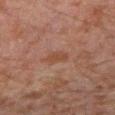{
  "biopsy_status": "not biopsied; imaged during a skin examination",
  "patient": {
    "sex": "male",
    "age_approx": 30
  },
  "lesion_size": {
    "long_diameter_mm_approx": 2.5
  },
  "lighting": "cross-polarized",
  "automated_metrics": {
    "area_mm2_approx": 4.5,
    "eccentricity": 0.5,
    "shape_asymmetry": 0.3,
    "cielab_L": 38,
    "cielab_a": 18,
    "cielab_b": 25,
    "vs_skin_darker_L": 5.0,
    "vs_skin_contrast_norm": 5.0,
    "color_variation_0_10": 2.0,
    "peripheral_color_asymmetry": 0.5,
    "lesion_detection_confidence_0_100": 100
  },
  "image": {
    "source": "total-body photography crop",
    "field_of_view_mm": 15
  },
  "site": "right forearm"
}